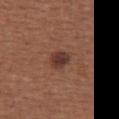Clinical impression:
The lesion was tiled from a total-body skin photograph and was not biopsied.
Acquisition and patient details:
Longest diameter approximately 2.5 mm. A roughly 15 mm field-of-view crop from a total-body skin photograph. The lesion is located on the chest. A female subject, aged 53 to 57.